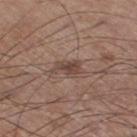The lesion was tiled from a total-body skin photograph and was not biopsied.
An algorithmic analysis of the crop reported a lesion color around L≈44 a*≈17 b*≈23 in CIELAB and roughly 9 lightness units darker than nearby skin.
Imaged with white-light lighting.
Measured at roughly 3.5 mm in maximum diameter.
The patient is a male about 60 years old.
A region of skin cropped from a whole-body photographic capture, roughly 15 mm wide.
From the right thigh.